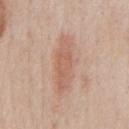Acquisition and patient details:
A close-up tile cropped from a whole-body skin photograph, about 15 mm across. A male subject, roughly 60 years of age. From the chest. Measured at roughly 7 mm in maximum diameter. The lesion-visualizer software estimated an area of roughly 13 mm², an outline eccentricity of about 0.95 (0 = round, 1 = elongated), and a symmetry-axis asymmetry near 0.2. It also reported border irregularity of about 4 on a 0–10 scale and peripheral color asymmetry of about 0.5. The software also gave a nevus-likeness score of about 25/100.